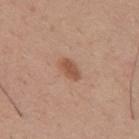{"biopsy_status": "not biopsied; imaged during a skin examination", "lesion_size": {"long_diameter_mm_approx": 3.0}, "image": {"source": "total-body photography crop", "field_of_view_mm": 15}, "patient": {"sex": "male", "age_approx": 30}, "site": "mid back"}